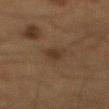Imaged during a routine full-body skin examination; the lesion was not biopsied and no histopathology is available.
Longest diameter approximately 2.5 mm.
A male patient in their mid- to late 60s.
This is a cross-polarized tile.
Automated tile analysis of the lesion measured an average lesion color of about L≈27 a*≈13 b*≈23 (CIELAB) and about 6 CIELAB-L* units darker than the surrounding skin. And it measured border irregularity of about 2.5 on a 0–10 scale and a color-variation rating of about 2/10.
From the mid back.
A 15 mm crop from a total-body photograph taken for skin-cancer surveillance.